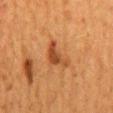Recorded during total-body skin imaging; not selected for excision or biopsy. A close-up tile cropped from a whole-body skin photograph, about 15 mm across. The lesion is located on the mid back. The lesion-visualizer software estimated a mean CIELAB color near L≈40 a*≈24 b*≈34, a lesion–skin lightness drop of about 9, and a normalized border contrast of about 7.5. The software also gave a nevus-likeness score of about 15/100 and a detector confidence of about 100 out of 100 that the crop contains a lesion. A female subject about 50 years old. Longest diameter approximately 4 mm. Imaged with cross-polarized lighting.